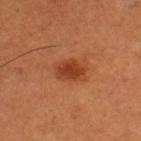Q: Was this lesion biopsied?
A: catalogued during a skin exam; not biopsied
Q: Where on the body is the lesion?
A: the left thigh
Q: What did automated image analysis measure?
A: a footprint of about 8 mm²; roughly 10 lightness units darker than nearby skin and a normalized lesion–skin contrast near 7.5; border irregularity of about 1.5 on a 0–10 scale, internal color variation of about 3 on a 0–10 scale, and radial color variation of about 1; a classifier nevus-likeness of about 100/100 and lesion-presence confidence of about 100/100
Q: How large is the lesion?
A: ~4 mm (longest diameter)
Q: Illumination type?
A: cross-polarized
Q: Patient demographics?
A: male, roughly 50 years of age
Q: What is the imaging modality?
A: ~15 mm crop, total-body skin-cancer survey